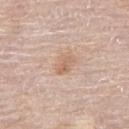notes: catalogued during a skin exam; not biopsied
location: the right upper arm
imaging modality: 15 mm crop, total-body photography
patient: female, aged approximately 65